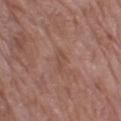Assessment:
Captured during whole-body skin photography for melanoma surveillance; the lesion was not biopsied.
Image and clinical context:
Imaged with white-light lighting. Longest diameter approximately 2.5 mm. An algorithmic analysis of the crop reported a lesion area of about 2.5 mm² and an eccentricity of roughly 0.9. And it measured a lesion color around L≈48 a*≈21 b*≈26 in CIELAB and about 6 CIELAB-L* units darker than the surrounding skin. And it measured a border-irregularity index near 3.5/10, internal color variation of about 0.5 on a 0–10 scale, and a peripheral color-asymmetry measure near 0. The software also gave an automated nevus-likeness rating near 0 out of 100 and a lesion-detection confidence of about 100/100. On the right thigh. This image is a 15 mm lesion crop taken from a total-body photograph. A female patient aged around 70.